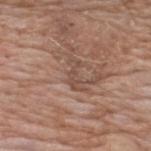Impression:
The lesion was tiled from a total-body skin photograph and was not biopsied.
Image and clinical context:
An algorithmic analysis of the crop reported a lesion color around L≈49 a*≈18 b*≈26 in CIELAB, roughly 7 lightness units darker than nearby skin, and a normalized lesion–skin contrast near 5.5. It also reported a border-irregularity index near 7/10, a within-lesion color-variation index near 0.5/10, and a peripheral color-asymmetry measure near 0. On the upper back. A male subject aged 58–62. This is a white-light tile. A lesion tile, about 15 mm wide, cut from a 3D total-body photograph. Approximately 4 mm at its widest.